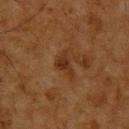{"biopsy_status": "not biopsied; imaged during a skin examination", "patient": {"sex": "male", "age_approx": 60}, "site": "upper back", "lighting": "cross-polarized", "image": {"source": "total-body photography crop", "field_of_view_mm": 15}}